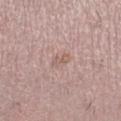Assessment: Captured during whole-body skin photography for melanoma surveillance; the lesion was not biopsied. Background: A lesion tile, about 15 mm wide, cut from a 3D total-body photograph. A female subject roughly 25 years of age. Located on the right lower leg. Automated image analysis of the tile measured a classifier nevus-likeness of about 0/100 and a lesion-detection confidence of about 100/100. The tile uses white-light illumination.